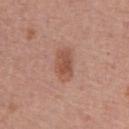| key | value |
|---|---|
| follow-up | total-body-photography surveillance lesion; no biopsy |
| lesion size | ~4 mm (longest diameter) |
| body site | the chest |
| illumination | white-light illumination |
| image | ~15 mm crop, total-body skin-cancer survey |
| subject | male, approximately 65 years of age |
| automated metrics | a lesion color around L≈52 a*≈23 b*≈29 in CIELAB, about 9 CIELAB-L* units darker than the surrounding skin, and a normalized lesion–skin contrast near 7; a peripheral color-asymmetry measure near 1; a classifier nevus-likeness of about 75/100 |